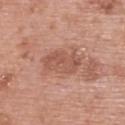Impression: No biopsy was performed on this lesion — it was imaged during a full skin examination and was not determined to be concerning. Clinical summary: This is a white-light tile. A 15 mm crop from a total-body photograph taken for skin-cancer surveillance. Approximately 5 mm at its widest. On the upper back. The subject is a female aged 58–62.